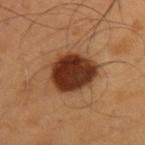Notes:
• follow-up · total-body-photography surveillance lesion; no biopsy
• image source · total-body-photography crop, ~15 mm field of view
• anatomic site · the back
• patient · male, aged 53 to 57